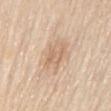Imaged during a routine full-body skin examination; the lesion was not biopsied and no histopathology is available.
Imaged with white-light lighting.
Cropped from a whole-body photographic skin survey; the tile spans about 15 mm.
A female subject, aged 68–72.
On the back.
Approximately 3 mm at its widest.
The total-body-photography lesion software estimated a lesion color around L≈66 a*≈17 b*≈33 in CIELAB and a lesion–skin lightness drop of about 8. The analysis additionally found border irregularity of about 4 on a 0–10 scale, a color-variation rating of about 3/10, and peripheral color asymmetry of about 1. It also reported a lesion-detection confidence of about 80/100.